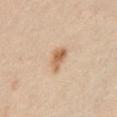No biopsy was performed on this lesion — it was imaged during a full skin examination and was not determined to be concerning.
The subject is a male aged 63–67.
A close-up tile cropped from a whole-body skin photograph, about 15 mm across.
The lesion is on the abdomen.
The tile uses cross-polarized illumination.
About 3.5 mm across.